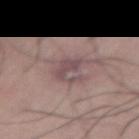Q: Was a biopsy performed?
A: no biopsy performed (imaged during a skin exam)
Q: Who is the patient?
A: male, aged 53 to 57
Q: Illumination type?
A: white-light
Q: Lesion location?
A: the abdomen
Q: How large is the lesion?
A: about 4 mm
Q: What is the imaging modality?
A: ~15 mm crop, total-body skin-cancer survey